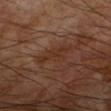workup: no biopsy performed (imaged during a skin exam) | anatomic site: the right forearm | subject: male, aged 68–72 | lesion diameter: about 4.5 mm | lighting: cross-polarized illumination | TBP lesion metrics: a border-irregularity index near 5/10 and radial color variation of about 0.5 | image source: ~15 mm crop, total-body skin-cancer survey.